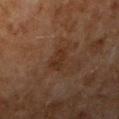Imaged during a routine full-body skin examination; the lesion was not biopsied and no histopathology is available. A region of skin cropped from a whole-body photographic capture, roughly 15 mm wide. The lesion is located on the right upper arm. An algorithmic analysis of the crop reported an area of roughly 5 mm², an eccentricity of roughly 0.85, and two-axis asymmetry of about 0.3. The analysis additionally found a lesion color around L≈26 a*≈17 b*≈25 in CIELAB, a lesion–skin lightness drop of about 5, and a normalized border contrast of about 6. It also reported a border-irregularity rating of about 3/10, internal color variation of about 2.5 on a 0–10 scale, and a peripheral color-asymmetry measure near 1. And it measured a classifier nevus-likeness of about 5/100. The recorded lesion diameter is about 3.5 mm. Captured under cross-polarized illumination. A female patient approximately 60 years of age.